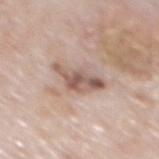biopsy_status: not biopsied; imaged during a skin examination
site: back
image:
  source: total-body photography crop
  field_of_view_mm: 15
lighting: white-light
automated_metrics:
  area_mm2_approx: 9.5
  eccentricity: 0.85
  nevus_likeness_0_100: 0
lesion_size:
  long_diameter_mm_approx: 5.0
patient:
  sex: male
  age_approx: 80